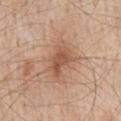Clinical impression:
Part of a total-body skin-imaging series; this lesion was reviewed on a skin check and was not flagged for biopsy.
Image and clinical context:
A male subject, about 60 years old. Imaged with white-light lighting. The lesion-visualizer software estimated a lesion color around L≈55 a*≈21 b*≈31 in CIELAB, about 10 CIELAB-L* units darker than the surrounding skin, and a normalized border contrast of about 7. And it measured a border-irregularity rating of about 4.5/10, internal color variation of about 3.5 on a 0–10 scale, and radial color variation of about 1.5. It also reported a nevus-likeness score of about 45/100 and a lesion-detection confidence of about 100/100. About 4 mm across. From the mid back. This image is a 15 mm lesion crop taken from a total-body photograph.